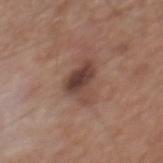notes — no biopsy performed (imaged during a skin exam); patient — male, about 65 years old; acquisition — 15 mm crop, total-body photography; anatomic site — the mid back.